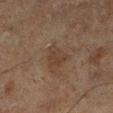{"biopsy_status": "not biopsied; imaged during a skin examination", "lighting": "cross-polarized", "lesion_size": {"long_diameter_mm_approx": 3.0}, "site": "left lower leg", "automated_metrics": {"cielab_L": 30, "cielab_a": 13, "cielab_b": 22, "vs_skin_darker_L": 5.0, "lesion_detection_confidence_0_100": 100}, "image": {"source": "total-body photography crop", "field_of_view_mm": 15}, "patient": {"sex": "male", "age_approx": 75}}